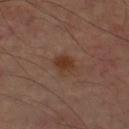biopsy_status: not biopsied; imaged during a skin examination
automated_metrics:
  area_mm2_approx: 5.0
  eccentricity: 0.65
  shape_asymmetry: 0.2
  cielab_L: 34
  cielab_a: 19
  cielab_b: 27
  vs_skin_darker_L: 7.0
  vs_skin_contrast_norm: 8.0
  border_irregularity_0_10: 2.0
  color_variation_0_10: 2.0
  peripheral_color_asymmetry: 0.5
  nevus_likeness_0_100: 80
  lesion_detection_confidence_0_100: 100
patient:
  sex: male
  age_approx: 60
image:
  source: total-body photography crop
  field_of_view_mm: 15
site: left upper arm
lesion_size:
  long_diameter_mm_approx: 2.5